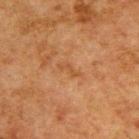follow-up: total-body-photography surveillance lesion; no biopsy | body site: the upper back | patient: male, in their 50s | image: ~15 mm tile from a whole-body skin photo | illumination: cross-polarized | TBP lesion metrics: a lesion color around L≈42 a*≈20 b*≈33 in CIELAB, a lesion–skin lightness drop of about 5, and a lesion-to-skin contrast of about 5 (normalized; higher = more distinct).